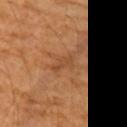Notes:
* biopsy status — no biopsy performed (imaged during a skin exam)
* image source — total-body-photography crop, ~15 mm field of view
* diameter — ≈3 mm
* image-analysis metrics — a border-irregularity index near 3.5/10, a within-lesion color-variation index near 1/10, and a peripheral color-asymmetry measure near 0.5; lesion-presence confidence of about 95/100
* anatomic site — the arm
* patient — male, about 60 years old
* tile lighting — cross-polarized